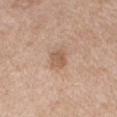Clinical impression:
No biopsy was performed on this lesion — it was imaged during a full skin examination and was not determined to be concerning.
Clinical summary:
The patient is a female in their mid-70s. Cropped from a whole-body photographic skin survey; the tile spans about 15 mm. The lesion is on the right upper arm. The tile uses white-light illumination.